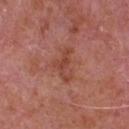Clinical impression: Imaged during a routine full-body skin examination; the lesion was not biopsied and no histopathology is available. Clinical summary: A male patient, aged approximately 65. An algorithmic analysis of the crop reported an average lesion color of about L≈45 a*≈27 b*≈30 (CIELAB) and a lesion-to-skin contrast of about 6.5 (normalized; higher = more distinct). It also reported border irregularity of about 8 on a 0–10 scale, a color-variation rating of about 1/10, and peripheral color asymmetry of about 0.5. The recorded lesion diameter is about 4 mm. On the chest. A close-up tile cropped from a whole-body skin photograph, about 15 mm across. Imaged with white-light lighting.